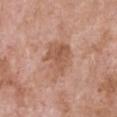| field | value |
|---|---|
| notes | total-body-photography surveillance lesion; no biopsy |
| size | about 5 mm |
| imaging modality | ~15 mm crop, total-body skin-cancer survey |
| automated metrics | a mean CIELAB color near L≈56 a*≈22 b*≈30, about 9 CIELAB-L* units darker than the surrounding skin, and a normalized border contrast of about 6.5 |
| patient | female, aged 73–77 |
| body site | the front of the torso |
| lighting | white-light illumination |